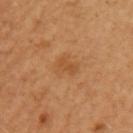No biopsy was performed on this lesion — it was imaged during a full skin examination and was not determined to be concerning.